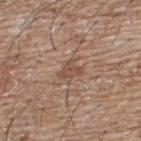{"biopsy_status": "not biopsied; imaged during a skin examination", "site": "upper back", "image": {"source": "total-body photography crop", "field_of_view_mm": 15}, "patient": {"sex": "male", "age_approx": 65}}